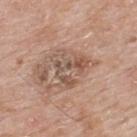Cropped from a total-body skin-imaging series; the visible field is about 15 mm. The subject is a male aged 63–67. Automated tile analysis of the lesion measured an outline eccentricity of about 0.45 (0 = round, 1 = elongated) and a shape-asymmetry score of about 0.7 (0 = symmetric). This is a white-light tile. About 4.5 mm across. On the upper back.